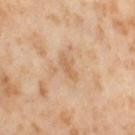biopsy status: no biopsy performed (imaged during a skin exam)
tile lighting: cross-polarized
lesion size: about 3 mm
imaging modality: ~15 mm tile from a whole-body skin photo
automated lesion analysis: a lesion area of about 3 mm², an eccentricity of roughly 0.9, and two-axis asymmetry of about 0.3; a lesion color around L≈63 a*≈19 b*≈37 in CIELAB, about 7 CIELAB-L* units darker than the surrounding skin, and a normalized border contrast of about 5; border irregularity of about 3.5 on a 0–10 scale, a within-lesion color-variation index near 0.5/10, and radial color variation of about 0; an automated nevus-likeness rating near 0 out of 100 and a detector confidence of about 100 out of 100 that the crop contains a lesion
subject: female, aged around 55
site: the left thigh